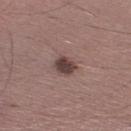Q: Patient demographics?
A: male, aged around 35
Q: What is the anatomic site?
A: the leg
Q: What is the imaging modality?
A: ~15 mm crop, total-body skin-cancer survey